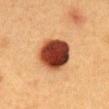notes: no biopsy performed (imaged during a skin exam)
body site: the chest
lesion size: about 4.5 mm
automated lesion analysis: an average lesion color of about L≈34 a*≈25 b*≈29 (CIELAB) and a normalized border contrast of about 18; an automated nevus-likeness rating near 100 out of 100 and a detector confidence of about 100 out of 100 that the crop contains a lesion
image: total-body-photography crop, ~15 mm field of view
patient: female, aged approximately 40
illumination: cross-polarized